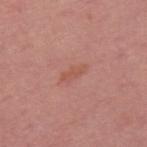Imaged during a routine full-body skin examination; the lesion was not biopsied and no histopathology is available.
A male subject in their mid- to late 50s.
From the upper back.
Imaged with white-light lighting.
A 15 mm close-up tile from a total-body photography series done for melanoma screening.